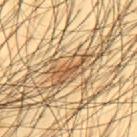location = the mid back
diameter = ~4.5 mm (longest diameter)
image = ~15 mm crop, total-body skin-cancer survey
illumination = cross-polarized
subject = male, aged approximately 45